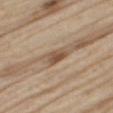Case summary:
* notes · imaged on a skin check; not biopsied
* image · total-body-photography crop, ~15 mm field of view
* tile lighting · white-light illumination
* subject · male, in their 70s
* automated metrics · an average lesion color of about L≈52 a*≈16 b*≈29 (CIELAB), a lesion–skin lightness drop of about 11, and a normalized border contrast of about 8; a border-irregularity index near 2/10, a color-variation rating of about 3/10, and peripheral color asymmetry of about 1
* anatomic site · the right thigh